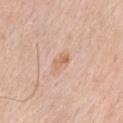workup: no biopsy performed (imaged during a skin exam)
location: the chest
lesion size: ~3 mm (longest diameter)
lighting: white-light
acquisition: 15 mm crop, total-body photography
subject: male, about 80 years old